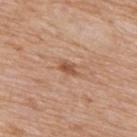On the back. Approximately 2.5 mm at its widest. The subject is a male aged 58 to 62. A close-up tile cropped from a whole-body skin photograph, about 15 mm across. Imaged with white-light lighting. The total-body-photography lesion software estimated an average lesion color of about L≈54 a*≈22 b*≈33 (CIELAB), a lesion–skin lightness drop of about 10, and a normalized border contrast of about 7. The analysis additionally found a within-lesion color-variation index near 1.5/10 and a peripheral color-asymmetry measure near 0.5. And it measured a classifier nevus-likeness of about 30/100.